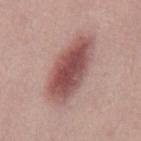Q: Is there a histopathology result?
A: catalogued during a skin exam; not biopsied
Q: Who is the patient?
A: male, aged approximately 30
Q: What is the imaging modality?
A: ~15 mm crop, total-body skin-cancer survey